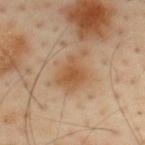patient: male, aged around 55 | anatomic site: the back | image source: ~15 mm crop, total-body skin-cancer survey.